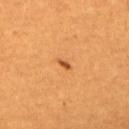Notes:
– workup — no biopsy performed (imaged during a skin exam)
– image-analysis metrics — a lesion color around L≈50 a*≈27 b*≈43 in CIELAB, a lesion–skin lightness drop of about 12, and a lesion-to-skin contrast of about 8 (normalized; higher = more distinct); an automated nevus-likeness rating near 95 out of 100 and a detector confidence of about 100 out of 100 that the crop contains a lesion
– anatomic site — the upper back
– tile lighting — cross-polarized
– image source — ~15 mm tile from a whole-body skin photo
– patient — male, aged 58 to 62